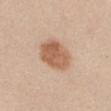* follow-up · total-body-photography surveillance lesion; no biopsy
* patient · female, aged around 30
* acquisition · ~15 mm crop, total-body skin-cancer survey
* automated metrics · a lesion area of about 14 mm², an outline eccentricity of about 0.7 (0 = round, 1 = elongated), and a symmetry-axis asymmetry near 0.15; an automated nevus-likeness rating near 100 out of 100 and lesion-presence confidence of about 100/100
* lesion diameter · ≈5 mm
* lighting · white-light illumination
* body site · the back